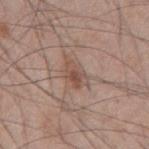The lesion was photographed on a routine skin check and not biopsied; there is no pathology result.
Longest diameter approximately 4 mm.
A 15 mm close-up tile from a total-body photography series done for melanoma screening.
From the back.
A male patient aged around 45.